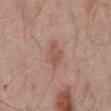The lesion was photographed on a routine skin check and not biopsied; there is no pathology result. Automated tile analysis of the lesion measured an average lesion color of about L≈53 a*≈22 b*≈27 (CIELAB) and a normalized lesion–skin contrast near 6. It also reported a border-irregularity index near 2.5/10 and internal color variation of about 1.5 on a 0–10 scale. About 3.5 mm across. The lesion is located on the abdomen. Captured under white-light illumination. A 15 mm crop from a total-body photograph taken for skin-cancer surveillance. The patient is a male about 70 years old.